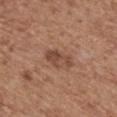Impression: Imaged during a routine full-body skin examination; the lesion was not biopsied and no histopathology is available. Image and clinical context: A 15 mm crop from a total-body photograph taken for skin-cancer surveillance. The lesion is located on the back. Automated image analysis of the tile measured a footprint of about 6.5 mm² and two-axis asymmetry of about 0.25. The software also gave an automated nevus-likeness rating near 25 out of 100 and lesion-presence confidence of about 100/100. The lesion's longest dimension is about 3.5 mm. The patient is a male in their mid- to late 60s. Imaged with white-light lighting.